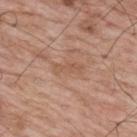The lesion was tiled from a total-body skin photograph and was not biopsied.
The recorded lesion diameter is about 3.5 mm.
Captured under white-light illumination.
A close-up tile cropped from a whole-body skin photograph, about 15 mm across.
On the upper back.
The patient is a male in their 70s.